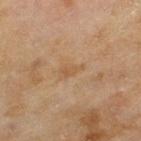Findings:
* follow-up — total-body-photography surveillance lesion; no biopsy
* illumination — cross-polarized
* subject — female, aged approximately 60
* image — ~15 mm tile from a whole-body skin photo
* image-analysis metrics — a lesion area of about 3 mm², an eccentricity of roughly 0.85, and a shape-asymmetry score of about 0.4 (0 = symmetric); a lesion color around L≈50 a*≈17 b*≈33 in CIELAB, roughly 6 lightness units darker than nearby skin, and a lesion-to-skin contrast of about 5 (normalized; higher = more distinct); a border-irregularity rating of about 4/10, a color-variation rating of about 1/10, and a peripheral color-asymmetry measure near 0.5; a classifier nevus-likeness of about 0/100 and a lesion-detection confidence of about 100/100
* lesion diameter — ~3 mm (longest diameter)
* anatomic site — the left thigh